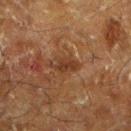Clinical impression:
Captured during whole-body skin photography for melanoma surveillance; the lesion was not biopsied.
Background:
Located on the left lower leg. The subject is a male aged around 60. A 15 mm crop from a total-body photograph taken for skin-cancer surveillance. Imaged with cross-polarized lighting. Longest diameter approximately 3.5 mm.